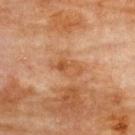Clinical impression:
The lesion was tiled from a total-body skin photograph and was not biopsied.
Acquisition and patient details:
The subject is a female roughly 80 years of age. The lesion is located on the upper back. This image is a 15 mm lesion crop taken from a total-body photograph.